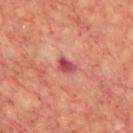Impression: The lesion was photographed on a routine skin check and not biopsied; there is no pathology result. Clinical summary: The patient is a male aged around 65. The lesion's longest dimension is about 2.5 mm. The lesion is on the upper back. This image is a 15 mm lesion crop taken from a total-body photograph. The tile uses cross-polarized illumination.